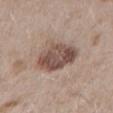Findings:
* notes — no biopsy performed (imaged during a skin exam)
* patient — male, in their mid-50s
* location — the right upper arm
* acquisition — total-body-photography crop, ~15 mm field of view